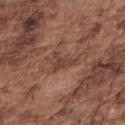| key | value |
|---|---|
| patient | male, aged approximately 75 |
| body site | the right upper arm |
| acquisition | total-body-photography crop, ~15 mm field of view |
| tile lighting | white-light illumination |